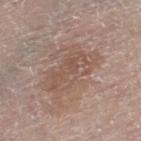workup = catalogued during a skin exam; not biopsied | tile lighting = white-light | lesion diameter = ~7 mm (longest diameter) | image = 15 mm crop, total-body photography | anatomic site = the left thigh | subject = male, roughly 80 years of age.